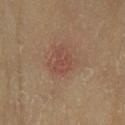  biopsy_status: not biopsied; imaged during a skin examination
  automated_metrics:
    eccentricity: 0.75
    shape_asymmetry: 0.45
    nevus_likeness_0_100: 5
    lesion_detection_confidence_0_100: 100
  lesion_size:
    long_diameter_mm_approx: 3.5
  image:
    source: total-body photography crop
    field_of_view_mm: 15
  site: left lower leg
  lighting: cross-polarized
  patient:
    sex: male
    age_approx: 65